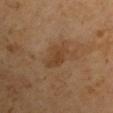Image and clinical context: A male patient roughly 65 years of age. A close-up tile cropped from a whole-body skin photograph, about 15 mm across. On the left arm. Approximately 4 mm at its widest. The tile uses cross-polarized illumination.